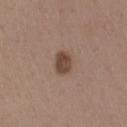  biopsy_status: not biopsied; imaged during a skin examination
  lesion_size:
    long_diameter_mm_approx: 2.5
  automated_metrics:
    cielab_L: 44
    cielab_a: 17
    cielab_b: 26
    vs_skin_darker_L: 12.0
    nevus_likeness_0_100: 80
  lighting: white-light
  image:
    source: total-body photography crop
    field_of_view_mm: 15
  site: arm
  patient:
    sex: male
    age_approx: 40